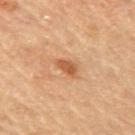Recorded during total-body skin imaging; not selected for excision or biopsy. A female subject about 65 years old. Cropped from a total-body skin-imaging series; the visible field is about 15 mm. On the right upper arm. An algorithmic analysis of the crop reported a lesion color around L≈57 a*≈25 b*≈39 in CIELAB and roughly 11 lightness units darker than nearby skin. The analysis additionally found an automated nevus-likeness rating near 50 out of 100. Measured at roughly 2.5 mm in maximum diameter. Imaged with cross-polarized lighting.